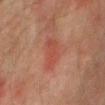biopsy status: total-body-photography surveillance lesion; no biopsy
lesion diameter: ≈4.5 mm
subject: male, in their mid- to late 70s
anatomic site: the chest
imaging modality: 15 mm crop, total-body photography